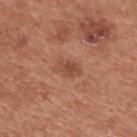Part of a total-body skin-imaging series; this lesion was reviewed on a skin check and was not flagged for biopsy.
The lesion is located on the upper back.
A close-up tile cropped from a whole-body skin photograph, about 15 mm across.
A male subject in their mid- to late 50s.